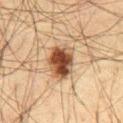Impression: This lesion was catalogued during total-body skin photography and was not selected for biopsy. Context: A 15 mm close-up extracted from a 3D total-body photography capture. On the front of the torso. The lesion-visualizer software estimated a lesion area of about 12 mm² and two-axis asymmetry of about 0.15. And it measured about 17 CIELAB-L* units darker than the surrounding skin and a normalized border contrast of about 12.5. The software also gave a nevus-likeness score of about 100/100 and a detector confidence of about 100 out of 100 that the crop contains a lesion. This is a cross-polarized tile. A male patient, aged 63 to 67.